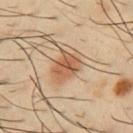Q: Was this lesion biopsied?
A: no biopsy performed (imaged during a skin exam)